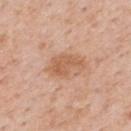| key | value |
|---|---|
| biopsy status | catalogued during a skin exam; not biopsied |
| body site | the back |
| tile lighting | white-light illumination |
| subject | male, aged 58 to 62 |
| image-analysis metrics | an area of roughly 9.5 mm², an eccentricity of roughly 0.85, and two-axis asymmetry of about 0.3; a lesion color around L≈60 a*≈22 b*≈33 in CIELAB, roughly 9 lightness units darker than nearby skin, and a lesion-to-skin contrast of about 6.5 (normalized; higher = more distinct) |
| imaging modality | ~15 mm tile from a whole-body skin photo |